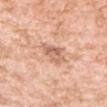<case>
  <biopsy_status>not biopsied; imaged during a skin examination</biopsy_status>
  <patient>
    <sex>female</sex>
    <age_approx>40</age_approx>
  </patient>
  <lesion_size>
    <long_diameter_mm_approx>3.5</long_diameter_mm_approx>
  </lesion_size>
  <image>
    <source>total-body photography crop</source>
    <field_of_view_mm>15</field_of_view_mm>
  </image>
  <site>right forearm</site>
  <lighting>white-light</lighting>
</case>